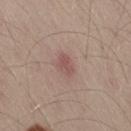The lesion was photographed on a routine skin check and not biopsied; there is no pathology result. A male patient about 55 years old. A 15 mm crop from a total-body photograph taken for skin-cancer surveillance. From the right thigh. The total-body-photography lesion software estimated a classifier nevus-likeness of about 5/100 and a lesion-detection confidence of about 100/100. Imaged with white-light lighting.